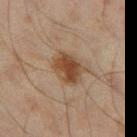notes: total-body-photography surveillance lesion; no biopsy
patient: male, roughly 45 years of age
image: total-body-photography crop, ~15 mm field of view
lesion diameter: about 4 mm
illumination: cross-polarized
location: the left thigh
automated metrics: roughly 9 lightness units darker than nearby skin and a normalized lesion–skin contrast near 9; border irregularity of about 2 on a 0–10 scale; a detector confidence of about 100 out of 100 that the crop contains a lesion